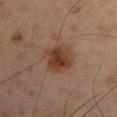Impression:
No biopsy was performed on this lesion — it was imaged during a full skin examination and was not determined to be concerning.
Image and clinical context:
Located on the left upper arm. A male patient, aged 48–52. A lesion tile, about 15 mm wide, cut from a 3D total-body photograph. Automated tile analysis of the lesion measured a footprint of about 10 mm², a shape eccentricity near 0.55, and two-axis asymmetry of about 0.15. The analysis additionally found internal color variation of about 4 on a 0–10 scale. It also reported an automated nevus-likeness rating near 95 out of 100 and lesion-presence confidence of about 100/100.